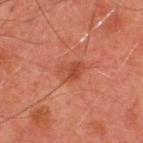follow-up = catalogued during a skin exam; not biopsied | site = the upper back | image = ~15 mm tile from a whole-body skin photo | diameter = ≈3 mm | patient = male, approximately 45 years of age | tile lighting = cross-polarized illumination.